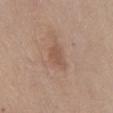biopsy_status: not biopsied; imaged during a skin examination
image:
  source: total-body photography crop
  field_of_view_mm: 15
site: abdomen
lighting: white-light
patient:
  sex: male
  age_approx: 55